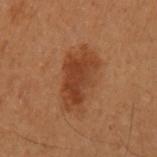| key | value |
|---|---|
| notes | total-body-photography surveillance lesion; no biopsy |
| patient | female, aged around 50 |
| location | the right upper arm |
| automated metrics | an average lesion color of about L≈35 a*≈21 b*≈30 (CIELAB), roughly 8 lightness units darker than nearby skin, and a normalized border contrast of about 7.5; a color-variation rating of about 3.5/10 and peripheral color asymmetry of about 1; a classifier nevus-likeness of about 80/100 and a detector confidence of about 100 out of 100 that the crop contains a lesion |
| lighting | cross-polarized illumination |
| image source | 15 mm crop, total-body photography |